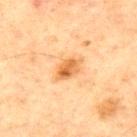Captured during whole-body skin photography for melanoma surveillance; the lesion was not biopsied. An algorithmic analysis of the crop reported a footprint of about 5.5 mm² and two-axis asymmetry of about 0.15. The software also gave an average lesion color of about L≈54 a*≈22 b*≈39 (CIELAB), about 11 CIELAB-L* units darker than the surrounding skin, and a normalized border contrast of about 8.5. The analysis additionally found a classifier nevus-likeness of about 80/100 and a detector confidence of about 100 out of 100 that the crop contains a lesion. A male subject about 65 years old. A roughly 15 mm field-of-view crop from a total-body skin photograph. The lesion's longest dimension is about 3.5 mm. Captured under cross-polarized illumination. On the chest.